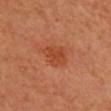Case summary:
• workup — catalogued during a skin exam; not biopsied
• lesion size — about 3 mm
• subject — female, in their mid-50s
• location — the head or neck
• imaging modality — total-body-photography crop, ~15 mm field of view